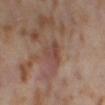Captured during whole-body skin photography for melanoma surveillance; the lesion was not biopsied. The lesion's longest dimension is about 3 mm. The lesion is located on the leg. Captured under cross-polarized illumination. Automated image analysis of the tile measured a nevus-likeness score of about 0/100 and a detector confidence of about 100 out of 100 that the crop contains a lesion. A close-up tile cropped from a whole-body skin photograph, about 15 mm across. A female subject, about 55 years old.